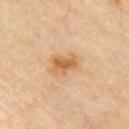Measured at roughly 3.5 mm in maximum diameter. The lesion is on the chest. The lesion-visualizer software estimated an area of roughly 7 mm², an eccentricity of roughly 0.75, and a shape-asymmetry score of about 0.25 (0 = symmetric). The analysis additionally found an average lesion color of about L≈64 a*≈21 b*≈41 (CIELAB) and roughly 11 lightness units darker than nearby skin. Imaged with cross-polarized lighting. A roughly 15 mm field-of-view crop from a total-body skin photograph.